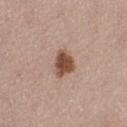Imaged during a routine full-body skin examination; the lesion was not biopsied and no histopathology is available. The lesion's longest dimension is about 3 mm. On the left thigh. A female subject, aged approximately 50. The tile uses white-light illumination. A region of skin cropped from a whole-body photographic capture, roughly 15 mm wide. The total-body-photography lesion software estimated a shape eccentricity near 0.4 and a symmetry-axis asymmetry near 0.25. And it measured roughly 15 lightness units darker than nearby skin.